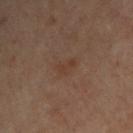<case>
  <patient>
    <sex>male</sex>
    <age_approx>50</age_approx>
  </patient>
  <site>upper back</site>
  <image>
    <source>total-body photography crop</source>
    <field_of_view_mm>15</field_of_view_mm>
  </image>
</case>